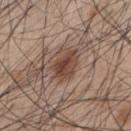Recorded during total-body skin imaging; not selected for excision or biopsy. The lesion's longest dimension is about 4 mm. Imaged with white-light lighting. A male subject aged around 45. A 15 mm close-up extracted from a 3D total-body photography capture. From the back.